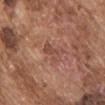- workup — no biopsy performed (imaged during a skin exam)
- lighting — white-light
- image-analysis metrics — a color-variation rating of about 4/10 and a peripheral color-asymmetry measure near 1.5; a classifier nevus-likeness of about 0/100
- subject — male, aged approximately 75
- size — about 3 mm
- anatomic site — the chest
- acquisition — ~15 mm crop, total-body skin-cancer survey